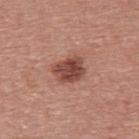Part of a total-body skin-imaging series; this lesion was reviewed on a skin check and was not flagged for biopsy. Cropped from a total-body skin-imaging series; the visible field is about 15 mm. The subject is a male aged 38–42. The lesion is on the upper back.